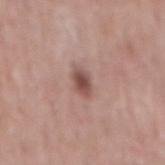follow-up: no biopsy performed (imaged during a skin exam); diameter: ≈3 mm; subject: male, aged 48–52; image source: ~15 mm tile from a whole-body skin photo; location: the mid back.